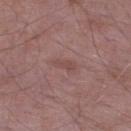Recorded during total-body skin imaging; not selected for excision or biopsy. A close-up tile cropped from a whole-body skin photograph, about 15 mm across. The lesion is on the right lower leg. This is a white-light tile. Approximately 3 mm at its widest. Automated tile analysis of the lesion measured an area of roughly 3.5 mm², an outline eccentricity of about 0.9 (0 = round, 1 = elongated), and a symmetry-axis asymmetry near 0.3. The analysis additionally found a color-variation rating of about 0.5/10 and peripheral color asymmetry of about 0. The patient is a male aged approximately 50.